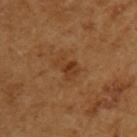From the left upper arm. A roughly 15 mm field-of-view crop from a total-body skin photograph. A female subject aged 53 to 57. The lesion's longest dimension is about 3 mm. Captured under cross-polarized illumination.